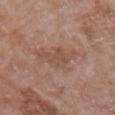Notes:
- workup: no biopsy performed (imaged during a skin exam)
- patient: female, aged around 70
- site: the left upper arm
- acquisition: ~15 mm crop, total-body skin-cancer survey